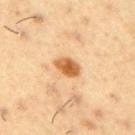Clinical impression: Imaged during a routine full-body skin examination; the lesion was not biopsied and no histopathology is available. Background: The patient is a male aged approximately 55. From the right upper arm. Imaged with cross-polarized lighting. A 15 mm close-up tile from a total-body photography series done for melanoma screening. About 3.5 mm across. Automated tile analysis of the lesion measured a lesion area of about 5.5 mm², an outline eccentricity of about 0.75 (0 = round, 1 = elongated), and two-axis asymmetry of about 0.15.